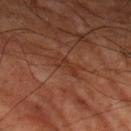biopsy status = total-body-photography surveillance lesion; no biopsy.